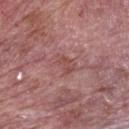Located on the back.
A male patient aged approximately 75.
Captured under white-light illumination.
A region of skin cropped from a whole-body photographic capture, roughly 15 mm wide.